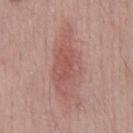Located on the front of the torso.
The total-body-photography lesion software estimated a lesion color around L≈54 a*≈24 b*≈24 in CIELAB, a lesion–skin lightness drop of about 9, and a lesion-to-skin contrast of about 6 (normalized; higher = more distinct).
Captured under white-light illumination.
Cropped from a whole-body photographic skin survey; the tile spans about 15 mm.
A male subject in their mid- to late 40s.